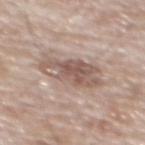| field | value |
|---|---|
| biopsy status | total-body-photography surveillance lesion; no biopsy |
| image source | total-body-photography crop, ~15 mm field of view |
| automated lesion analysis | an area of roughly 16 mm², a shape eccentricity near 0.85, and a symmetry-axis asymmetry near 0.2; an average lesion color of about L≈56 a*≈16 b*≈24 (CIELAB), roughly 11 lightness units darker than nearby skin, and a normalized border contrast of about 7; a border-irregularity index near 3/10 and a within-lesion color-variation index near 6/10 |
| tile lighting | white-light |
| site | the mid back |
| subject | male, approximately 80 years of age |
| size | about 6 mm |